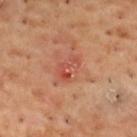notes — imaged on a skin check; not biopsied
diameter — ~3.5 mm (longest diameter)
anatomic site — the back
patient — male, aged 53 to 57
imaging modality — 15 mm crop, total-body photography
illumination — cross-polarized